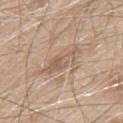biopsy status=catalogued during a skin exam; not biopsied
patient=male, about 80 years old
site=the back
lesion size=~5.5 mm (longest diameter)
image=15 mm crop, total-body photography
illumination=white-light illumination